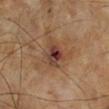follow-up: catalogued during a skin exam; not biopsied
size: ~3 mm (longest diameter)
subject: male, in their mid- to late 60s
image: ~15 mm crop, total-body skin-cancer survey
anatomic site: the left lower leg